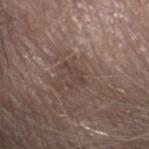Part of a total-body skin-imaging series; this lesion was reviewed on a skin check and was not flagged for biopsy. The tile uses white-light illumination. A region of skin cropped from a whole-body photographic capture, roughly 15 mm wide. The patient is a male aged around 30. Measured at roughly 3 mm in maximum diameter. Automated tile analysis of the lesion measured a nevus-likeness score of about 0/100 and lesion-presence confidence of about 85/100.